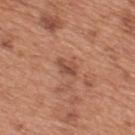Q: Is there a histopathology result?
A: catalogued during a skin exam; not biopsied
Q: How was this image acquired?
A: ~15 mm tile from a whole-body skin photo
Q: How was the tile lit?
A: white-light illumination
Q: Patient demographics?
A: male, approximately 65 years of age
Q: What is the anatomic site?
A: the mid back
Q: Automated lesion metrics?
A: an average lesion color of about L≈49 a*≈24 b*≈30 (CIELAB) and a normalized border contrast of about 7
Q: Lesion size?
A: ~2.5 mm (longest diameter)